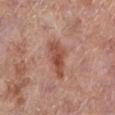This lesion was catalogued during total-body skin photography and was not selected for biopsy. From the leg. A female patient roughly 75 years of age. The tile uses white-light illumination. An algorithmic analysis of the crop reported a classifier nevus-likeness of about 25/100 and a lesion-detection confidence of about 100/100. A lesion tile, about 15 mm wide, cut from a 3D total-body photograph. Approximately 5 mm at its widest.